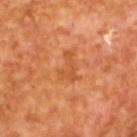{
  "biopsy_status": "not biopsied; imaged during a skin examination",
  "image": {
    "source": "total-body photography crop",
    "field_of_view_mm": 15
  },
  "patient": {
    "sex": "male",
    "age_approx": 65
  },
  "lighting": "cross-polarized",
  "lesion_size": {
    "long_diameter_mm_approx": 3.5
  }
}